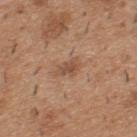Clinical impression:
No biopsy was performed on this lesion — it was imaged during a full skin examination and was not determined to be concerning.
Background:
A male subject about 40 years old. On the upper back. A region of skin cropped from a whole-body photographic capture, roughly 15 mm wide.